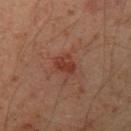  biopsy_status: not biopsied; imaged during a skin examination
  lesion_size:
    long_diameter_mm_approx: 3.0
  site: left upper arm
  patient:
    sex: male
    age_approx: 50
  lighting: cross-polarized
  automated_metrics:
    area_mm2_approx: 5.0
    shape_asymmetry: 0.2
    vs_skin_darker_L: 7.0
    vs_skin_contrast_norm: 7.0
    nevus_likeness_0_100: 65
    lesion_detection_confidence_0_100: 100
  image:
    source: total-body photography crop
    field_of_view_mm: 15